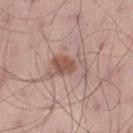workup: no biopsy performed (imaged during a skin exam) | site: the left thigh | patient: male, aged 53 to 57 | imaging modality: total-body-photography crop, ~15 mm field of view | lighting: white-light | automated lesion analysis: a footprint of about 9.5 mm², an outline eccentricity of about 0.85 (0 = round, 1 = elongated), and two-axis asymmetry of about 0.4; an average lesion color of about L≈55 a*≈20 b*≈24 (CIELAB), roughly 10 lightness units darker than nearby skin, and a lesion-to-skin contrast of about 7 (normalized; higher = more distinct).